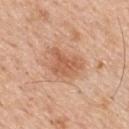Assessment: Recorded during total-body skin imaging; not selected for excision or biopsy. Image and clinical context: The tile uses white-light illumination. The lesion is located on the mid back. This image is a 15 mm lesion crop taken from a total-body photograph. An algorithmic analysis of the crop reported a lesion color around L≈59 a*≈24 b*≈34 in CIELAB. The analysis additionally found a border-irregularity rating of about 4/10, a color-variation rating of about 2.5/10, and radial color variation of about 1. A male patient, roughly 60 years of age.